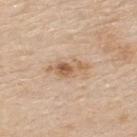Imaged during a routine full-body skin examination; the lesion was not biopsied and no histopathology is available.
The total-body-photography lesion software estimated an area of roughly 7 mm², an eccentricity of roughly 0.9, and two-axis asymmetry of about 0.25. The analysis additionally found a mean CIELAB color near L≈61 a*≈17 b*≈33, about 10 CIELAB-L* units darker than the surrounding skin, and a normalized lesion–skin contrast near 7. The software also gave a within-lesion color-variation index near 6.5/10 and radial color variation of about 2. And it measured a classifier nevus-likeness of about 50/100 and a detector confidence of about 100 out of 100 that the crop contains a lesion.
A male patient aged 78–82.
A close-up tile cropped from a whole-body skin photograph, about 15 mm across.
On the upper back.
Approximately 5 mm at its widest.